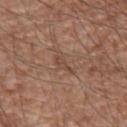This lesion was catalogued during total-body skin photography and was not selected for biopsy. The tile uses white-light illumination. The patient is a male approximately 60 years of age. The total-body-photography lesion software estimated an area of roughly 3.5 mm², an eccentricity of roughly 0.85, and two-axis asymmetry of about 0.5. The analysis additionally found a lesion color around L≈46 a*≈19 b*≈27 in CIELAB, about 6 CIELAB-L* units darker than the surrounding skin, and a normalized border contrast of about 5. The software also gave a within-lesion color-variation index near 1.5/10 and a peripheral color-asymmetry measure near 0.5. Cropped from a total-body skin-imaging series; the visible field is about 15 mm. From the right upper arm.